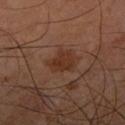Captured during whole-body skin photography for melanoma surveillance; the lesion was not biopsied. Automated image analysis of the tile measured about 7 CIELAB-L* units darker than the surrounding skin and a normalized lesion–skin contrast near 7.5. The analysis additionally found an automated nevus-likeness rating near 75 out of 100 and a detector confidence of about 100 out of 100 that the crop contains a lesion. This image is a 15 mm lesion crop taken from a total-body photograph. The tile uses cross-polarized illumination. Located on the right forearm. The subject is a male about 60 years old.